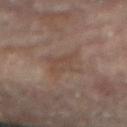<case>
  <biopsy_status>not biopsied; imaged during a skin examination</biopsy_status>
  <site>leg</site>
  <automated_metrics>
    <cielab_L>41</cielab_L>
    <cielab_a>14</cielab_a>
    <cielab_b>22</cielab_b>
    <vs_skin_contrast_norm>4.5</vs_skin_contrast_norm>
    <border_irregularity_0_10>5.0</border_irregularity_0_10>
    <peripheral_color_asymmetry>0.5</peripheral_color_asymmetry>
    <nevus_likeness_0_100>0</nevus_likeness_0_100>
  </automated_metrics>
  <lesion_size>
    <long_diameter_mm_approx>4.5</long_diameter_mm_approx>
  </lesion_size>
  <image>
    <source>total-body photography crop</source>
    <field_of_view_mm>15</field_of_view_mm>
  </image>
  <patient>
    <sex>female</sex>
    <age_approx>80</age_approx>
  </patient>
  <lighting>cross-polarized</lighting>
</case>